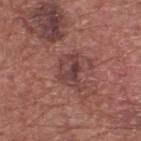biopsy status: catalogued during a skin exam; not biopsied | illumination: white-light illumination | automated lesion analysis: a lesion area of about 8 mm², a shape eccentricity near 0.7, and a symmetry-axis asymmetry near 0.35; a lesion-detection confidence of about 100/100 | image: 15 mm crop, total-body photography | anatomic site: the upper back | patient: male, roughly 75 years of age.